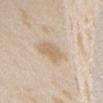Imaged during a routine full-body skin examination; the lesion was not biopsied and no histopathology is available. Cropped from a whole-body photographic skin survey; the tile spans about 15 mm. A female patient, in their mid-20s. This is a white-light tile. The lesion-visualizer software estimated a lesion area of about 7 mm², an outline eccentricity of about 0.75 (0 = round, 1 = elongated), and a symmetry-axis asymmetry near 0.3. It also reported a mean CIELAB color near L≈66 a*≈13 b*≈32, roughly 8 lightness units darker than nearby skin, and a lesion-to-skin contrast of about 6 (normalized; higher = more distinct). The software also gave lesion-presence confidence of about 100/100. From the head or neck.